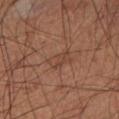Imaged during a routine full-body skin examination; the lesion was not biopsied and no histopathology is available. A close-up tile cropped from a whole-body skin photograph, about 15 mm across. The lesion-visualizer software estimated an eccentricity of roughly 0.85 and a shape-asymmetry score of about 0.3 (0 = symmetric). A male patient about 60 years old. On the left lower leg. Imaged with cross-polarized lighting. Longest diameter approximately 3 mm.